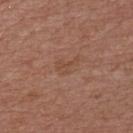| key | value |
|---|---|
| biopsy status | catalogued during a skin exam; not biopsied |
| patient | male, in their mid- to late 50s |
| anatomic site | the front of the torso |
| imaging modality | ~15 mm tile from a whole-body skin photo |